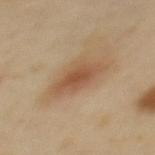{"biopsy_status": "not biopsied; imaged during a skin examination", "patient": {"sex": "female", "age_approx": 40}, "image": {"source": "total-body photography crop", "field_of_view_mm": 15}, "site": "upper back"}